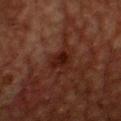This is a cross-polarized tile.
A male subject aged 58 to 62.
Approximately 2.5 mm at its widest.
Automated tile analysis of the lesion measured a lesion area of about 3.5 mm², a shape eccentricity near 0.75, and a symmetry-axis asymmetry near 0.25. The analysis additionally found a mean CIELAB color near L≈16 a*≈20 b*≈20 and a normalized border contrast of about 10. The software also gave an automated nevus-likeness rating near 5 out of 100 and a detector confidence of about 100 out of 100 that the crop contains a lesion.
A close-up tile cropped from a whole-body skin photograph, about 15 mm across.
The lesion is on the front of the torso.